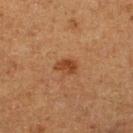| field | value |
|---|---|
| subject | male, aged approximately 75 |
| illumination | cross-polarized |
| site | the right lower leg |
| acquisition | total-body-photography crop, ~15 mm field of view |
| size | ≈2.5 mm |